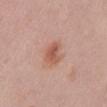<record>
  <biopsy_status>not biopsied; imaged during a skin examination</biopsy_status>
  <patient>
    <sex>male</sex>
    <age_approx>60</age_approx>
  </patient>
  <site>front of the torso</site>
  <image>
    <source>total-body photography crop</source>
    <field_of_view_mm>15</field_of_view_mm>
  </image>
  <lighting>white-light</lighting>
</record>